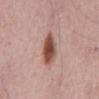Case summary:
– notes: total-body-photography surveillance lesion; no biopsy
– TBP lesion metrics: a mean CIELAB color near L≈50 a*≈22 b*≈26 and a normalized border contrast of about 11
– subject: male, aged 63 to 67
– lighting: white-light
– size: ≈4 mm
– anatomic site: the mid back
– image source: ~15 mm crop, total-body skin-cancer survey